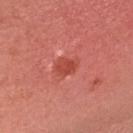Clinical impression:
The lesion was photographed on a routine skin check and not biopsied; there is no pathology result.
Clinical summary:
A roughly 15 mm field-of-view crop from a total-body skin photograph. This is a white-light tile. The lesion's longest dimension is about 2.5 mm. The patient is a male aged 43–47. On the head or neck. Automated image analysis of the tile measured a lesion area of about 5 mm², an outline eccentricity of about 0.65 (0 = round, 1 = elongated), and a shape-asymmetry score of about 0.3 (0 = symmetric). The analysis additionally found a nevus-likeness score of about 55/100 and lesion-presence confidence of about 100/100.